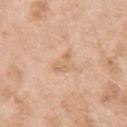Clinical summary:
From the left upper arm. Automated image analysis of the tile measured about 7 CIELAB-L* units darker than the surrounding skin and a lesion-to-skin contrast of about 5 (normalized; higher = more distinct). It also reported a nevus-likeness score of about 0/100 and lesion-presence confidence of about 100/100. A region of skin cropped from a whole-body photographic capture, roughly 15 mm wide. A female patient, aged around 75. Approximately 3 mm at its widest.